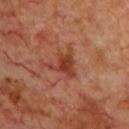Q: Is there a histopathology result?
A: no biopsy performed (imaged during a skin exam)
Q: What did automated image analysis measure?
A: a classifier nevus-likeness of about 5/100
Q: Lesion size?
A: about 4 mm
Q: How was the tile lit?
A: cross-polarized
Q: Lesion location?
A: the chest
Q: How was this image acquired?
A: 15 mm crop, total-body photography
Q: Who is the patient?
A: male, aged approximately 70